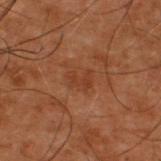Image and clinical context: A lesion tile, about 15 mm wide, cut from a 3D total-body photograph. This is a cross-polarized tile. The total-body-photography lesion software estimated a nevus-likeness score of about 0/100 and a lesion-detection confidence of about 100/100. About 2.5 mm across. Located on the upper back. The patient is a male aged approximately 50.